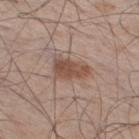Assessment: Imaged during a routine full-body skin examination; the lesion was not biopsied and no histopathology is available. Background: From the right thigh. A male patient aged 58–62. Automated image analysis of the tile measured internal color variation of about 3 on a 0–10 scale and radial color variation of about 1. The analysis additionally found a nevus-likeness score of about 90/100. The tile uses white-light illumination. A region of skin cropped from a whole-body photographic capture, roughly 15 mm wide. Longest diameter approximately 4 mm.